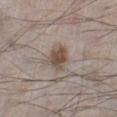Assessment: No biopsy was performed on this lesion — it was imaged during a full skin examination and was not determined to be concerning. Clinical summary: Located on the left lower leg. A male patient in their 70s. A lesion tile, about 15 mm wide, cut from a 3D total-body photograph.